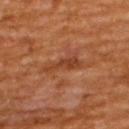The lesion was tiled from a total-body skin photograph and was not biopsied.
Longest diameter approximately 4.5 mm.
A region of skin cropped from a whole-body photographic capture, roughly 15 mm wide.
From the upper back.
The patient is a male in their mid-60s.